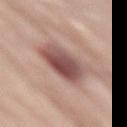The lesion was photographed on a routine skin check and not biopsied; there is no pathology result.
A male subject aged 73–77.
From the lower back.
A region of skin cropped from a whole-body photographic capture, roughly 15 mm wide.